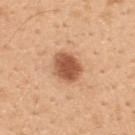lesion size: ≈3.5 mm | lighting: white-light | subject: male, aged 48 to 52 | image source: ~15 mm tile from a whole-body skin photo | location: the back.